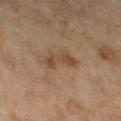<case>
<image>
  <source>total-body photography crop</source>
  <field_of_view_mm>15</field_of_view_mm>
</image>
<lighting>cross-polarized</lighting>
<automated_metrics>
  <cielab_L>45</cielab_L>
  <cielab_a>17</cielab_a>
  <cielab_b>31</cielab_b>
</automated_metrics>
<patient>
  <sex>male</sex>
  <age_approx>65</age_approx>
</patient>
<lesion_size>
  <long_diameter_mm_approx>4.0</long_diameter_mm_approx>
</lesion_size>
</case>